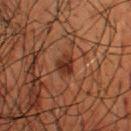subject=male, in their 60s | lesion size=≈3 mm | anatomic site=the head or neck | lighting=cross-polarized | image=15 mm crop, total-body photography.